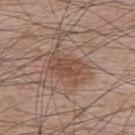workup — total-body-photography surveillance lesion; no biopsy
tile lighting — white-light
imaging modality — total-body-photography crop, ~15 mm field of view
subject — male, aged around 65
location — the upper back